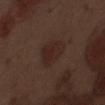Assessment: This lesion was catalogued during total-body skin photography and was not selected for biopsy. Background: The recorded lesion diameter is about 4 mm. This is a white-light tile. A roughly 15 mm field-of-view crop from a total-body skin photograph. A male patient aged around 70. On the front of the torso.